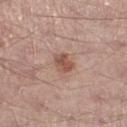On the left lower leg. Cropped from a whole-body photographic skin survey; the tile spans about 15 mm. A male patient in their 40s. The lesion-visualizer software estimated an area of roughly 4.5 mm², an outline eccentricity of about 0.7 (0 = round, 1 = elongated), and a symmetry-axis asymmetry near 0.25. It also reported a border-irregularity rating of about 2/10, a within-lesion color-variation index near 3/10, and radial color variation of about 1. The analysis additionally found a nevus-likeness score of about 70/100 and a detector confidence of about 100 out of 100 that the crop contains a lesion.